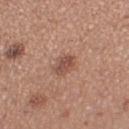This lesion was catalogued during total-body skin photography and was not selected for biopsy. A female patient about 30 years old. On the left thigh. A lesion tile, about 15 mm wide, cut from a 3D total-body photograph.